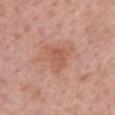notes = catalogued during a skin exam; not biopsied
acquisition = 15 mm crop, total-body photography
illumination = white-light
patient = female, aged 63 to 67
location = the upper back